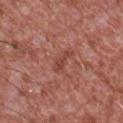Q: Was a biopsy performed?
A: total-body-photography surveillance lesion; no biopsy
Q: Lesion size?
A: ~3 mm (longest diameter)
Q: What kind of image is this?
A: total-body-photography crop, ~15 mm field of view
Q: Lesion location?
A: the chest
Q: What are the patient's age and sex?
A: male, aged around 45
Q: How was the tile lit?
A: white-light illumination